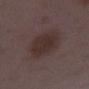Assessment: No biopsy was performed on this lesion — it was imaged during a full skin examination and was not determined to be concerning. Image and clinical context: Automated image analysis of the tile measured a shape eccentricity near 0.7 and a shape-asymmetry score of about 0.1 (0 = symmetric). The software also gave a mean CIELAB color near L≈30 a*≈14 b*≈17, a lesion–skin lightness drop of about 7, and a normalized lesion–skin contrast near 7.5. The analysis additionally found internal color variation of about 2.5 on a 0–10 scale. Imaged with white-light lighting. This image is a 15 mm lesion crop taken from a total-body photograph. From the right lower leg. A female subject, aged 28 to 32.